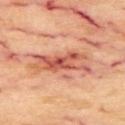{"biopsy_status": "not biopsied; imaged during a skin examination", "image": {"source": "total-body photography crop", "field_of_view_mm": 15}, "patient": {"sex": "male", "age_approx": 70}, "site": "upper back"}